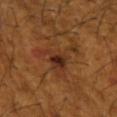Assessment: Imaged during a routine full-body skin examination; the lesion was not biopsied and no histopathology is available. Image and clinical context: About 6.5 mm across. A male subject, aged approximately 65. A roughly 15 mm field-of-view crop from a total-body skin photograph. An algorithmic analysis of the crop reported about 8 CIELAB-L* units darker than the surrounding skin and a normalized lesion–skin contrast near 8. The analysis additionally found a border-irregularity index near 9/10, internal color variation of about 7 on a 0–10 scale, and radial color variation of about 2. Located on the left upper arm.